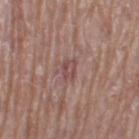The lesion was tiled from a total-body skin photograph and was not biopsied. A 15 mm close-up tile from a total-body photography series done for melanoma screening. The patient is a female roughly 60 years of age. From the left thigh. Captured under white-light illumination.